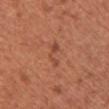biopsy status=no biopsy performed (imaged during a skin exam); body site=the chest; tile lighting=white-light illumination; lesion diameter=about 3 mm; subject=female, about 50 years old; imaging modality=total-body-photography crop, ~15 mm field of view; image-analysis metrics=a mean CIELAB color near L≈48 a*≈26 b*≈32, roughly 7 lightness units darker than nearby skin, and a normalized border contrast of about 5.5.